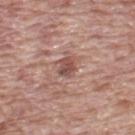biopsy status: imaged on a skin check; not biopsied
body site: the upper back
size: about 2.5 mm
tile lighting: white-light
patient: male, aged around 70
image source: ~15 mm crop, total-body skin-cancer survey